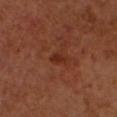follow-up: imaged on a skin check; not biopsied
tile lighting: cross-polarized illumination
imaging modality: total-body-photography crop, ~15 mm field of view
subject: male, roughly 50 years of age
body site: the head or neck
lesion diameter: ~2.5 mm (longest diameter)